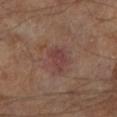Measured at roughly 3 mm in maximum diameter.
Located on the left lower leg.
An algorithmic analysis of the crop reported a lesion color around L≈38 a*≈22 b*≈21 in CIELAB, roughly 6 lightness units darker than nearby skin, and a normalized lesion–skin contrast near 6. The software also gave a nevus-likeness score of about 0/100 and lesion-presence confidence of about 100/100.
Cropped from a total-body skin-imaging series; the visible field is about 15 mm.
Captured under cross-polarized illumination.
A male subject, approximately 70 years of age.